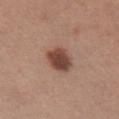  biopsy_status: not biopsied; imaged during a skin examination
  patient:
    sex: female
    age_approx: 55
  site: chest
  image:
    source: total-body photography crop
    field_of_view_mm: 15
  lighting: white-light
  lesion_size:
    long_diameter_mm_approx: 3.5